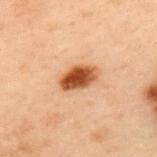No biopsy was performed on this lesion — it was imaged during a full skin examination and was not determined to be concerning. An algorithmic analysis of the crop reported a lesion–skin lightness drop of about 17 and a normalized lesion–skin contrast near 12.5. The analysis additionally found a peripheral color-asymmetry measure near 2. A male subject aged approximately 55. A roughly 15 mm field-of-view crop from a total-body skin photograph. About 4 mm across. The lesion is on the upper back.